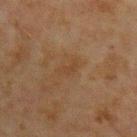| field | value |
|---|---|
| biopsy status | catalogued during a skin exam; not biopsied |
| image | ~15 mm crop, total-body skin-cancer survey |
| anatomic site | the chest |
| size | about 3 mm |
| patient | male, aged approximately 65 |
| tile lighting | cross-polarized |